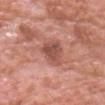| field | value |
|---|---|
| notes | no biopsy performed (imaged during a skin exam) |
| site | the head or neck |
| diameter | ~4 mm (longest diameter) |
| imaging modality | total-body-photography crop, ~15 mm field of view |
| subject | male, approximately 80 years of age |